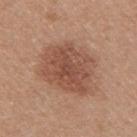Clinical impression: Captured during whole-body skin photography for melanoma surveillance; the lesion was not biopsied. Context: A region of skin cropped from a whole-body photographic capture, roughly 15 mm wide. Captured under white-light illumination. The lesion's longest dimension is about 7 mm. A female subject aged approximately 50. From the right upper arm. Automated image analysis of the tile measured a shape eccentricity near 0.65. And it measured a lesion–skin lightness drop of about 10 and a normalized border contrast of about 7.5. The analysis additionally found a border-irregularity rating of about 3/10, a color-variation rating of about 3.5/10, and peripheral color asymmetry of about 1. The analysis additionally found a nevus-likeness score of about 60/100 and a detector confidence of about 100 out of 100 that the crop contains a lesion.